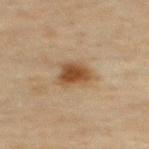Q: Is there a histopathology result?
A: imaged on a skin check; not biopsied
Q: What is the imaging modality?
A: ~15 mm crop, total-body skin-cancer survey
Q: What did automated image analysis measure?
A: a border-irregularity index near 3/10, a color-variation rating of about 3/10, and a peripheral color-asymmetry measure near 1; a nevus-likeness score of about 95/100 and lesion-presence confidence of about 100/100
Q: What is the anatomic site?
A: the upper back
Q: What lighting was used for the tile?
A: cross-polarized
Q: Patient demographics?
A: male, aged approximately 45
Q: How large is the lesion?
A: about 3.5 mm